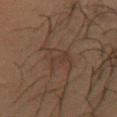Clinical impression:
The lesion was tiled from a total-body skin photograph and was not biopsied.
Context:
From the chest. The patient is a female roughly 20 years of age. Measured at roughly 6 mm in maximum diameter. Cropped from a total-body skin-imaging series; the visible field is about 15 mm.